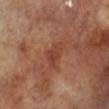Q: Was this lesion biopsied?
A: imaged on a skin check; not biopsied
Q: Lesion size?
A: ~4 mm (longest diameter)
Q: What is the imaging modality?
A: total-body-photography crop, ~15 mm field of view
Q: Where on the body is the lesion?
A: the left lower leg
Q: What did automated image analysis measure?
A: an area of roughly 6 mm², an outline eccentricity of about 0.8 (0 = round, 1 = elongated), and a symmetry-axis asymmetry near 0.45; an average lesion color of about L≈41 a*≈25 b*≈29 (CIELAB), roughly 7 lightness units darker than nearby skin, and a lesion-to-skin contrast of about 6 (normalized; higher = more distinct); a border-irregularity index near 4.5/10, internal color variation of about 3 on a 0–10 scale, and a peripheral color-asymmetry measure near 1; a classifier nevus-likeness of about 5/100 and lesion-presence confidence of about 100/100
Q: Patient demographics?
A: male, aged 68 to 72
Q: Illumination type?
A: cross-polarized